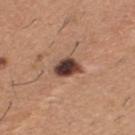Clinical impression:
The lesion was photographed on a routine skin check and not biopsied; there is no pathology result.
Context:
This is a white-light tile. From the upper back. Longest diameter approximately 3.5 mm. Cropped from a total-body skin-imaging series; the visible field is about 15 mm. The subject is a male in their 60s.